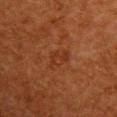A female subject in their 50s.
This is a cross-polarized tile.
A close-up tile cropped from a whole-body skin photograph, about 15 mm across.
An algorithmic analysis of the crop reported an automated nevus-likeness rating near 0 out of 100.
The lesion's longest dimension is about 3 mm.
From the chest.